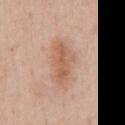Clinical impression:
The lesion was photographed on a routine skin check and not biopsied; there is no pathology result.
Background:
Approximately 5.5 mm at its widest. The patient is a male roughly 50 years of age. An algorithmic analysis of the crop reported a lesion–skin lightness drop of about 9 and a lesion-to-skin contrast of about 6.5 (normalized; higher = more distinct). And it measured a border-irregularity index near 3.5/10 and a color-variation rating of about 3.5/10. A 15 mm close-up tile from a total-body photography series done for melanoma screening. The lesion is located on the chest.